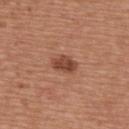Findings:
- TBP lesion metrics · an area of roughly 5.5 mm²; internal color variation of about 3 on a 0–10 scale; a nevus-likeness score of about 95/100 and a detector confidence of about 100 out of 100 that the crop contains a lesion
- imaging modality · ~15 mm crop, total-body skin-cancer survey
- lesion diameter · ~3 mm (longest diameter)
- subject · male, aged around 65
- site · the upper back